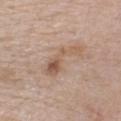Clinical impression: The lesion was tiled from a total-body skin photograph and was not biopsied. Context: A roughly 15 mm field-of-view crop from a total-body skin photograph. The recorded lesion diameter is about 6 mm. Located on the chest. A female patient, roughly 45 years of age. This is a white-light tile. An algorithmic analysis of the crop reported an automated nevus-likeness rating near 5 out of 100.